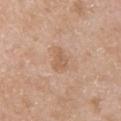Imaged during a routine full-body skin examination; the lesion was not biopsied and no histopathology is available. Located on the left upper arm. A female patient, aged 68–72. Imaged with white-light lighting. Cropped from a whole-body photographic skin survey; the tile spans about 15 mm. About 2.5 mm across. The total-body-photography lesion software estimated an average lesion color of about L≈60 a*≈19 b*≈32 (CIELAB), about 7 CIELAB-L* units darker than the surrounding skin, and a normalized lesion–skin contrast near 5.5.